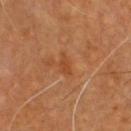notes: total-body-photography surveillance lesion; no biopsy
automated lesion analysis: a shape eccentricity near 0.85 and a symmetry-axis asymmetry near 0.4; a lesion–skin lightness drop of about 6 and a lesion-to-skin contrast of about 6 (normalized; higher = more distinct); border irregularity of about 3.5 on a 0–10 scale and radial color variation of about 0; a nevus-likeness score of about 0/100 and lesion-presence confidence of about 100/100
acquisition: 15 mm crop, total-body photography
body site: the chest
subject: male, aged approximately 60
lesion size: about 3 mm
tile lighting: cross-polarized